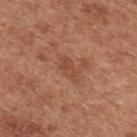Clinical impression: The lesion was photographed on a routine skin check and not biopsied; there is no pathology result. Context: The lesion is located on the upper back. This image is a 15 mm lesion crop taken from a total-body photograph. The lesion's longest dimension is about 3 mm. This is a white-light tile. The total-body-photography lesion software estimated a lesion–skin lightness drop of about 7 and a normalized border contrast of about 5. The analysis additionally found a border-irregularity rating of about 3.5/10, a color-variation rating of about 1.5/10, and radial color variation of about 0.5. The patient is a male aged 63 to 67.